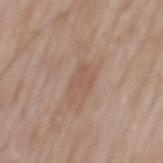Q: Was this lesion biopsied?
A: imaged on a skin check; not biopsied
Q: How was this image acquired?
A: 15 mm crop, total-body photography
Q: What is the anatomic site?
A: the mid back
Q: What are the patient's age and sex?
A: male, about 60 years old